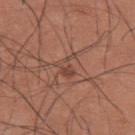The lesion was tiled from a total-body skin photograph and was not biopsied. A male patient, in their mid- to late 30s. The lesion is located on the upper back. The total-body-photography lesion software estimated a lesion area of about 4 mm² and an outline eccentricity of about 0.8 (0 = round, 1 = elongated). And it measured border irregularity of about 6 on a 0–10 scale and internal color variation of about 2.5 on a 0–10 scale. The analysis additionally found a nevus-likeness score of about 5/100 and lesion-presence confidence of about 90/100. The lesion's longest dimension is about 3 mm. A 15 mm close-up extracted from a 3D total-body photography capture.